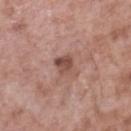The subject is a male in their mid-50s. From the leg. Captured under white-light illumination. Automated tile analysis of the lesion measured a border-irregularity index near 1.5/10, internal color variation of about 5 on a 0–10 scale, and a peripheral color-asymmetry measure near 2. The software also gave a nevus-likeness score of about 0/100. Longest diameter approximately 2.5 mm. Cropped from a whole-body photographic skin survey; the tile spans about 15 mm.